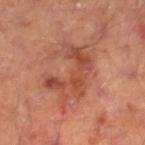Impression:
Imaged during a routine full-body skin examination; the lesion was not biopsied and no histopathology is available.
Acquisition and patient details:
Located on the right lower leg. A 15 mm close-up tile from a total-body photography series done for melanoma screening. Approximately 5.5 mm at its widest. The total-body-photography lesion software estimated an automated nevus-likeness rating near 0 out of 100. The tile uses cross-polarized illumination. A male subject, aged 63–67.